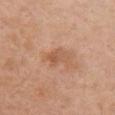workup=catalogued during a skin exam; not biopsied
site=the chest
size=about 3 mm
image source=15 mm crop, total-body photography
subject=female, in their mid- to late 60s
illumination=white-light